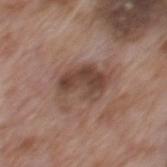Context:
Captured under white-light illumination. From the mid back. A male patient, aged 68 to 72. This image is a 15 mm lesion crop taken from a total-body photograph. About 6 mm across.